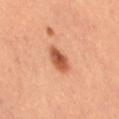Assessment:
Recorded during total-body skin imaging; not selected for excision or biopsy.
Context:
Automated tile analysis of the lesion measured a color-variation rating of about 5/10 and peripheral color asymmetry of about 1.5. A female patient, in their 60s. A region of skin cropped from a whole-body photographic capture, roughly 15 mm wide. Imaged with cross-polarized lighting. Located on the right thigh. The lesion's longest dimension is about 3.5 mm.